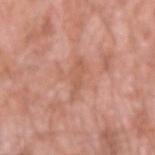Impression:
No biopsy was performed on this lesion — it was imaged during a full skin examination and was not determined to be concerning.
Image and clinical context:
Captured under white-light illumination. Located on the right upper arm. A 15 mm close-up extracted from a 3D total-body photography capture. The subject is a male aged 58 to 62. The lesion-visualizer software estimated an outline eccentricity of about 0.9 (0 = round, 1 = elongated) and a shape-asymmetry score of about 0.35 (0 = symmetric). It also reported an average lesion color of about L≈58 a*≈23 b*≈31 (CIELAB) and a normalized lesion–skin contrast near 5. About 4 mm across.